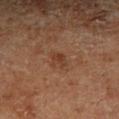Part of a total-body skin-imaging series; this lesion was reviewed on a skin check and was not flagged for biopsy. This is a cross-polarized tile. The total-body-photography lesion software estimated border irregularity of about 3 on a 0–10 scale. And it measured a classifier nevus-likeness of about 0/100 and a detector confidence of about 100 out of 100 that the crop contains a lesion. A male subject approximately 70 years of age. A 15 mm close-up tile from a total-body photography series done for melanoma screening. The lesion is located on the right lower leg.